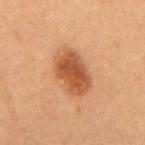Findings:
* follow-up · catalogued during a skin exam; not biopsied
* image source · ~15 mm tile from a whole-body skin photo
* location · the mid back
* subject · female, aged 58–62